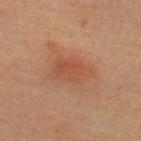follow-up = total-body-photography surveillance lesion; no biopsy
diameter = ~4.5 mm (longest diameter)
image source = 15 mm crop, total-body photography
lighting = cross-polarized illumination
patient = male, about 60 years old
site = the upper back
image-analysis metrics = an area of roughly 10 mm², a shape eccentricity near 0.8, and a symmetry-axis asymmetry near 0.25; a border-irregularity rating of about 2.5/10, a color-variation rating of about 2.5/10, and peripheral color asymmetry of about 0.5; a nevus-likeness score of about 50/100 and a detector confidence of about 100 out of 100 that the crop contains a lesion